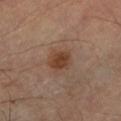No biopsy was performed on this lesion — it was imaged during a full skin examination and was not determined to be concerning. The tile uses cross-polarized illumination. Located on the right forearm. Cropped from a whole-body photographic skin survey; the tile spans about 15 mm. A male subject, approximately 55 years of age.